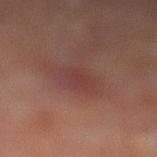Q: Was this lesion biopsied?
A: no biopsy performed (imaged during a skin exam)